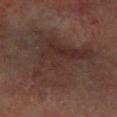Captured during whole-body skin photography for melanoma surveillance; the lesion was not biopsied. Located on the left forearm. The lesion-visualizer software estimated an average lesion color of about L≈31 a*≈15 b*≈19 (CIELAB) and a lesion–skin lightness drop of about 6. And it measured a border-irregularity index near 8.5/10 and a color-variation rating of about 5/10. The tile uses cross-polarized illumination. A region of skin cropped from a whole-body photographic capture, roughly 15 mm wide. The subject is a female aged 63 to 67. The recorded lesion diameter is about 11.5 mm.